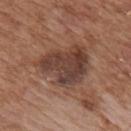Impression:
No biopsy was performed on this lesion — it was imaged during a full skin examination and was not determined to be concerning.
Clinical summary:
Measured at roughly 6.5 mm in maximum diameter. A roughly 15 mm field-of-view crop from a total-body skin photograph. Located on the mid back. A male patient, aged 63–67. The lesion-visualizer software estimated roughly 11 lightness units darker than nearby skin and a lesion-to-skin contrast of about 9 (normalized; higher = more distinct). It also reported a border-irregularity index near 4/10, a color-variation rating of about 5.5/10, and a peripheral color-asymmetry measure near 2. The analysis additionally found a nevus-likeness score of about 5/100.